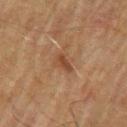Impression: No biopsy was performed on this lesion — it was imaged during a full skin examination and was not determined to be concerning. Clinical summary: On the arm. The tile uses cross-polarized illumination. Longest diameter approximately 2.5 mm. Cropped from a whole-body photographic skin survey; the tile spans about 15 mm. A male subject, aged approximately 75.